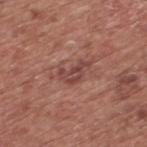| feature | finding |
|---|---|
| notes | catalogued during a skin exam; not biopsied |
| lighting | white-light illumination |
| lesion diameter | about 4 mm |
| image | total-body-photography crop, ~15 mm field of view |
| patient | male, aged 73–77 |
| anatomic site | the upper back |